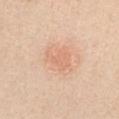Measured at roughly 4.5 mm in maximum diameter. A female patient aged 43 to 47. An algorithmic analysis of the crop reported a border-irregularity rating of about 3/10 and a within-lesion color-variation index near 2.5/10. The analysis additionally found an automated nevus-likeness rating near 35 out of 100. This is a white-light tile. A 15 mm close-up tile from a total-body photography series done for melanoma screening. Located on the right upper arm.